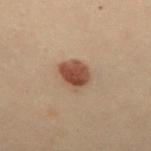* size · ≈3.5 mm
* tile lighting · cross-polarized illumination
* subject · female, roughly 30 years of age
* site · the mid back
* image · ~15 mm crop, total-body skin-cancer survey